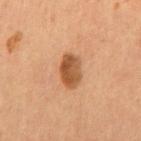patient:
  sex: male
  age_approx: 55
image:
  source: total-body photography crop
  field_of_view_mm: 15
lesion_size:
  long_diameter_mm_approx: 4.0
lighting: cross-polarized
site: abdomen